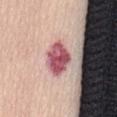Automated image analysis of the tile measured a lesion area of about 8 mm² and an eccentricity of roughly 0.7. On the front of the torso. The patient is a female roughly 55 years of age. A roughly 15 mm field-of-view crop from a total-body skin photograph.